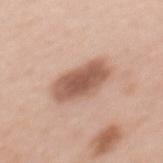Assessment:
No biopsy was performed on this lesion — it was imaged during a full skin examination and was not determined to be concerning.
Context:
This is a white-light tile. Automated tile analysis of the lesion measured an area of roughly 14 mm² and a symmetry-axis asymmetry near 0.15. And it measured a lesion color around L≈56 a*≈21 b*≈28 in CIELAB, about 14 CIELAB-L* units darker than the surrounding skin, and a normalized border contrast of about 9.5. The software also gave an automated nevus-likeness rating near 55 out of 100. A 15 mm crop from a total-body photograph taken for skin-cancer surveillance. A female patient aged 48 to 52. Longest diameter approximately 5.5 mm. The lesion is on the mid back.